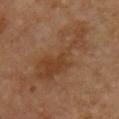Imaged during a routine full-body skin examination; the lesion was not biopsied and no histopathology is available. Imaged with cross-polarized lighting. A region of skin cropped from a whole-body photographic capture, roughly 15 mm wide. A male patient aged approximately 55. On the front of the torso. Automated image analysis of the tile measured a shape eccentricity near 0.95 and two-axis asymmetry of about 0.65. The recorded lesion diameter is about 9 mm.